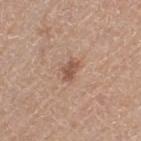This lesion was catalogued during total-body skin photography and was not selected for biopsy.
The patient is a female approximately 60 years of age.
An algorithmic analysis of the crop reported a lesion color around L≈53 a*≈20 b*≈27 in CIELAB, roughly 11 lightness units darker than nearby skin, and a normalized border contrast of about 7.5. The analysis additionally found a border-irregularity index near 2.5/10, a color-variation rating of about 2/10, and peripheral color asymmetry of about 1. The analysis additionally found a nevus-likeness score of about 25/100 and a lesion-detection confidence of about 100/100.
A 15 mm close-up extracted from a 3D total-body photography capture.
On the left thigh.